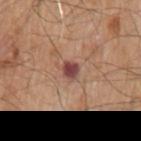Clinical impression: Recorded during total-body skin imaging; not selected for excision or biopsy. Clinical summary: The lesion is located on the left upper arm. Imaged with white-light lighting. A male subject about 80 years old. Measured at roughly 2.5 mm in maximum diameter. Cropped from a total-body skin-imaging series; the visible field is about 15 mm.